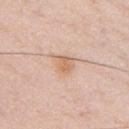automated lesion analysis — a lesion area of about 4.5 mm² and a shape eccentricity near 0.45; a within-lesion color-variation index near 2/10 and peripheral color asymmetry of about 0.5
location — the chest
patient — male, roughly 35 years of age
lighting — white-light illumination
image source — ~15 mm crop, total-body skin-cancer survey